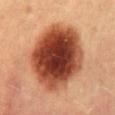Q: Was a biopsy performed?
A: imaged on a skin check; not biopsied
Q: What lighting was used for the tile?
A: cross-polarized illumination
Q: What is the anatomic site?
A: the mid back
Q: Who is the patient?
A: female, roughly 60 years of age
Q: How large is the lesion?
A: ≈8.5 mm
Q: What kind of image is this?
A: total-body-photography crop, ~15 mm field of view
Q: What did automated image analysis measure?
A: a border-irregularity index near 1.5/10, a color-variation rating of about 8/10, and a peripheral color-asymmetry measure near 2; a classifier nevus-likeness of about 100/100 and lesion-presence confidence of about 100/100